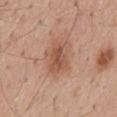notes: no biopsy performed (imaged during a skin exam) | location: the mid back | patient: male, in their mid- to late 50s | lesion size: ~4 mm (longest diameter) | image source: ~15 mm crop, total-body skin-cancer survey | TBP lesion metrics: a lesion area of about 8.5 mm² and a shape-asymmetry score of about 0.25 (0 = symmetric); an average lesion color of about L≈53 a*≈23 b*≈30 (CIELAB), roughly 10 lightness units darker than nearby skin, and a normalized lesion–skin contrast near 7; a lesion-detection confidence of about 100/100.